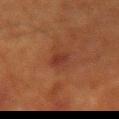biopsy_status: not biopsied; imaged during a skin examination
lighting: cross-polarized
site: right upper arm
lesion_size:
  long_diameter_mm_approx: 2.5
image:
  source: total-body photography crop
  field_of_view_mm: 15
patient:
  sex: male
  age_approx: 75